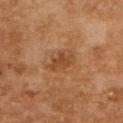Imaged during a routine full-body skin examination; the lesion was not biopsied and no histopathology is available. Captured under cross-polarized illumination. A female subject aged approximately 60. Approximately 4 mm at its widest. The lesion is located on the upper back. Cropped from a total-body skin-imaging series; the visible field is about 15 mm.